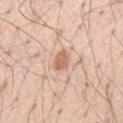Context: On the mid back. A roughly 15 mm field-of-view crop from a total-body skin photograph. The subject is a male in their mid-50s. Measured at roughly 2.5 mm in maximum diameter.